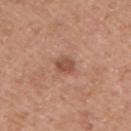Case summary:
• imaging modality · total-body-photography crop, ~15 mm field of view
• anatomic site · the upper back
• automated lesion analysis · a shape eccentricity near 0.75 and two-axis asymmetry of about 0.2; internal color variation of about 1.5 on a 0–10 scale and radial color variation of about 0.5; an automated nevus-likeness rating near 20 out of 100 and lesion-presence confidence of about 100/100
• tile lighting · white-light
• subject · male, about 55 years old